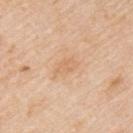The lesion was tiled from a total-body skin photograph and was not biopsied.
Approximately 3 mm at its widest.
The tile uses white-light illumination.
A close-up tile cropped from a whole-body skin photograph, about 15 mm across.
On the left upper arm.
The patient is a male about 75 years old.
Automated tile analysis of the lesion measured a footprint of about 4 mm² and an eccentricity of roughly 0.85. And it measured border irregularity of about 3.5 on a 0–10 scale, internal color variation of about 1.5 on a 0–10 scale, and peripheral color asymmetry of about 0.5.